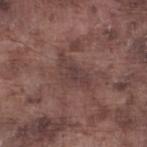Clinical impression: This lesion was catalogued during total-body skin photography and was not selected for biopsy. Image and clinical context: A male patient, about 75 years old. The lesion-visualizer software estimated a footprint of about 6.5 mm², an eccentricity of roughly 0.85, and a symmetry-axis asymmetry near 0.5. It also reported a lesion color around L≈38 a*≈18 b*≈18 in CIELAB, about 6 CIELAB-L* units darker than the surrounding skin, and a normalized lesion–skin contrast near 6. And it measured a within-lesion color-variation index near 2/10. From the left lower leg. Longest diameter approximately 4.5 mm. Imaged with white-light lighting. A 15 mm crop from a total-body photograph taken for skin-cancer surveillance.